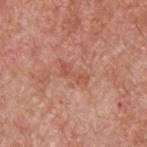biopsy status=imaged on a skin check; not biopsied